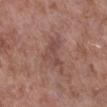notes = no biopsy performed (imaged during a skin exam) | image source = 15 mm crop, total-body photography | image-analysis metrics = a shape eccentricity near 0.7; a lesion color around L≈47 a*≈20 b*≈23 in CIELAB, a lesion–skin lightness drop of about 7, and a normalized lesion–skin contrast near 5.5; a nevus-likeness score of about 0/100 and a lesion-detection confidence of about 100/100 | site = the right lower leg | diameter = about 4 mm | lighting = white-light illumination | patient = male, about 55 years old.